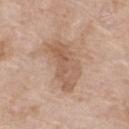follow-up = total-body-photography surveillance lesion; no biopsy
size = ~6.5 mm (longest diameter)
site = the right thigh
image = ~15 mm tile from a whole-body skin photo
subject = female, in their mid-70s
tile lighting = white-light